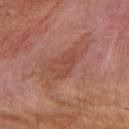Part of a total-body skin-imaging series; this lesion was reviewed on a skin check and was not flagged for biopsy. The lesion-visualizer software estimated a footprint of about 7.5 mm² and two-axis asymmetry of about 0.3. It also reported a lesion color around L≈47 a*≈26 b*≈29 in CIELAB and a normalized lesion–skin contrast near 5. The analysis additionally found a nevus-likeness score of about 0/100 and a detector confidence of about 90 out of 100 that the crop contains a lesion. A male subject, approximately 55 years of age. Measured at roughly 4 mm in maximum diameter. Located on the arm. Imaged with cross-polarized lighting. A 15 mm close-up tile from a total-body photography series done for melanoma screening.